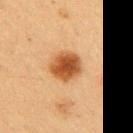Captured during whole-body skin photography for melanoma surveillance; the lesion was not biopsied.
A 15 mm close-up extracted from a 3D total-body photography capture.
Located on the arm.
The patient is a female aged around 40.